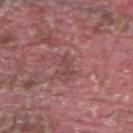This lesion was catalogued during total-body skin photography and was not selected for biopsy.
Imaged with white-light lighting.
A male patient, approximately 40 years of age.
On the right upper arm.
A close-up tile cropped from a whole-body skin photograph, about 15 mm across.
An algorithmic analysis of the crop reported a classifier nevus-likeness of about 0/100 and a lesion-detection confidence of about 80/100.